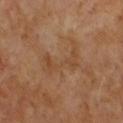The lesion's longest dimension is about 5.5 mm. This image is a 15 mm lesion crop taken from a total-body photograph. The subject is a male in their mid- to late 60s. The tile uses cross-polarized illumination. Automated tile analysis of the lesion measured a mean CIELAB color near L≈44 a*≈19 b*≈32, about 5 CIELAB-L* units darker than the surrounding skin, and a normalized border contrast of about 5. And it measured a border-irregularity rating of about 9/10, internal color variation of about 2 on a 0–10 scale, and a peripheral color-asymmetry measure near 0.5. And it measured a nevus-likeness score of about 0/100 and lesion-presence confidence of about 100/100.